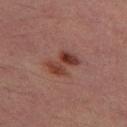Assessment: No biopsy was performed on this lesion — it was imaged during a full skin examination and was not determined to be concerning. Background: A close-up tile cropped from a whole-body skin photograph, about 15 mm across. The lesion's longest dimension is about 4 mm. Located on the left thigh. The patient is a male about 30 years old. The tile uses cross-polarized illumination.